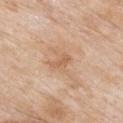- workup · imaged on a skin check; not biopsied
- image · total-body-photography crop, ~15 mm field of view
- lighting · white-light
- lesion diameter · ~2.5 mm (longest diameter)
- body site · the upper back
- automated lesion analysis · a lesion color around L≈60 a*≈20 b*≈35 in CIELAB, a lesion–skin lightness drop of about 8, and a normalized lesion–skin contrast near 5.5; border irregularity of about 4 on a 0–10 scale; a classifier nevus-likeness of about 0/100
- patient · male, in their mid-80s or older